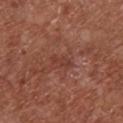Clinical summary:
This is a white-light tile. Longest diameter approximately 2.5 mm. The subject is a female aged approximately 75. The lesion is located on the chest. A 15 mm crop from a total-body photograph taken for skin-cancer surveillance.